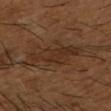{
  "site": "right forearm",
  "patient": {
    "sex": "male",
    "age_approx": 65
  },
  "lighting": "cross-polarized",
  "lesion_size": {
    "long_diameter_mm_approx": 6.5
  },
  "image": {
    "source": "total-body photography crop",
    "field_of_view_mm": 15
  }
}